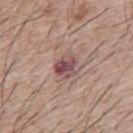Imaged during a routine full-body skin examination; the lesion was not biopsied and no histopathology is available. The lesion-visualizer software estimated an area of roughly 5.5 mm² and an outline eccentricity of about 0.55 (0 = round, 1 = elongated). The software also gave an automated nevus-likeness rating near 5 out of 100 and lesion-presence confidence of about 100/100. The lesion is located on the back. A male patient, in their mid-60s. A roughly 15 mm field-of-view crop from a total-body skin photograph. The tile uses white-light illumination.